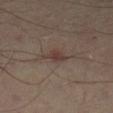Q: Was a biopsy performed?
A: imaged on a skin check; not biopsied